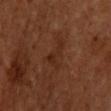The lesion was tiled from a total-body skin photograph and was not biopsied.
A male patient approximately 60 years of age.
A 15 mm close-up extracted from a 3D total-body photography capture.
The lesion is located on the upper back.
The tile uses cross-polarized illumination.
The recorded lesion diameter is about 3.5 mm.